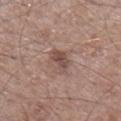Part of a total-body skin-imaging series; this lesion was reviewed on a skin check and was not flagged for biopsy.
Measured at roughly 3.5 mm in maximum diameter.
Automated image analysis of the tile measured internal color variation of about 3 on a 0–10 scale. The software also gave a detector confidence of about 100 out of 100 that the crop contains a lesion.
From the leg.
A male subject, approximately 65 years of age.
A region of skin cropped from a whole-body photographic capture, roughly 15 mm wide.
Captured under white-light illumination.